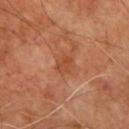<record>
<automated_metrics>
  <area_mm2_approx>4.5</area_mm2_approx>
  <eccentricity>0.55</eccentricity>
  <shape_asymmetry>0.2</shape_asymmetry>
  <border_irregularity_0_10>2.0</border_irregularity_0_10>
  <color_variation_0_10>2.5</color_variation_0_10>
  <nevus_likeness_0_100>0</nevus_likeness_0_100>
  <lesion_detection_confidence_0_100>100</lesion_detection_confidence_0_100>
</automated_metrics>
<patient>
  <sex>male</sex>
  <age_approx>65</age_approx>
</patient>
<lighting>cross-polarized</lighting>
<image>
  <source>total-body photography crop</source>
  <field_of_view_mm>15</field_of_view_mm>
</image>
</record>